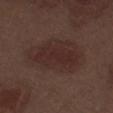biopsy status: no biopsy performed (imaged during a skin exam)
lighting: white-light illumination
image-analysis metrics: a border-irregularity rating of about 4/10 and peripheral color asymmetry of about 1; a classifier nevus-likeness of about 65/100 and a lesion-detection confidence of about 100/100
body site: the right thigh
image source: ~15 mm tile from a whole-body skin photo
subject: male, aged 68 to 72
size: ~5.5 mm (longest diameter)